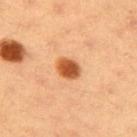  biopsy_status: not biopsied; imaged during a skin examination
  lighting: cross-polarized
  site: right upper arm
  lesion_size:
    long_diameter_mm_approx: 3.0
  automated_metrics:
    shape_asymmetry: 0.15
    cielab_L: 57
    cielab_a: 29
    cielab_b: 43
    border_irregularity_0_10: 1.5
    peripheral_color_asymmetry: 1.5
    nevus_likeness_0_100: 100
    lesion_detection_confidence_0_100: 100
  image:
    source: total-body photography crop
    field_of_view_mm: 15
  patient:
    sex: female
    age_approx: 35